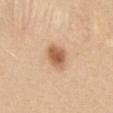Clinical impression: No biopsy was performed on this lesion — it was imaged during a full skin examination and was not determined to be concerning. Clinical summary: The tile uses white-light illumination. A female patient, aged 23 to 27. A lesion tile, about 15 mm wide, cut from a 3D total-body photograph. Located on the mid back.